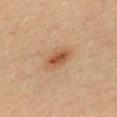biopsy status = catalogued during a skin exam; not biopsied
patient = female, aged 33 to 37
image source = total-body-photography crop, ~15 mm field of view
body site = the back